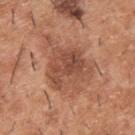image source: 15 mm crop, total-body photography
anatomic site: the upper back
patient: male, aged approximately 40